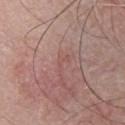{
  "patient": {
    "sex": "male",
    "age_approx": 45
  },
  "automated_metrics": {
    "area_mm2_approx": 1.5,
    "eccentricity": 0.85
  },
  "image": {
    "source": "total-body photography crop",
    "field_of_view_mm": 15
  },
  "site": "chest"
}